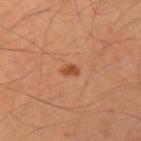Assessment: Captured during whole-body skin photography for melanoma surveillance; the lesion was not biopsied.